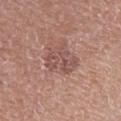notes — no biopsy performed (imaged during a skin exam)
image-analysis metrics — a footprint of about 8.5 mm², an eccentricity of roughly 0.8, and two-axis asymmetry of about 0.45; an average lesion color of about L≈51 a*≈21 b*≈24 (CIELAB), roughly 8 lightness units darker than nearby skin, and a normalized border contrast of about 6; a border-irregularity index near 6.5/10, internal color variation of about 3.5 on a 0–10 scale, and a peripheral color-asymmetry measure near 1.5
subject — female, aged 48 to 52
tile lighting — white-light
body site — the left upper arm
lesion size — ≈4.5 mm
image source — ~15 mm tile from a whole-body skin photo